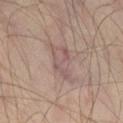| feature | finding |
|---|---|
| biopsy status | imaged on a skin check; not biopsied |
| body site | the right thigh |
| subject | male, aged approximately 45 |
| image | total-body-photography crop, ~15 mm field of view |